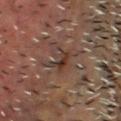Case summary:
- biopsy status — total-body-photography surveillance lesion; no biopsy
- imaging modality — ~15 mm crop, total-body skin-cancer survey
- patient — male, aged 53 to 57
- lesion diameter — ~4 mm (longest diameter)
- automated lesion analysis — an area of roughly 7 mm², an eccentricity of roughly 0.65, and a shape-asymmetry score of about 0.4 (0 = symmetric); an average lesion color of about L≈33 a*≈15 b*≈21 (CIELAB), about 7 CIELAB-L* units darker than the surrounding skin, and a normalized border contrast of about 6.5; a border-irregularity index near 6/10, internal color variation of about 6 on a 0–10 scale, and a peripheral color-asymmetry measure near 2
- site — the chest
- lighting — cross-polarized illumination